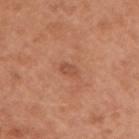Assessment: The lesion was photographed on a routine skin check and not biopsied; there is no pathology result. Background: The subject is a male in their mid-50s. From the arm. A 15 mm close-up extracted from a 3D total-body photography capture. About 2.5 mm across. Automated image analysis of the tile measured an average lesion color of about L≈51 a*≈26 b*≈33 (CIELAB) and a normalized border contrast of about 5.5. It also reported an automated nevus-likeness rating near 0 out of 100 and lesion-presence confidence of about 100/100.